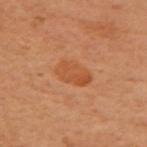Q: Was a biopsy performed?
A: total-body-photography surveillance lesion; no biopsy
Q: Where on the body is the lesion?
A: the arm
Q: What did automated image analysis measure?
A: a lesion area of about 7 mm² and two-axis asymmetry of about 0.25; an automated nevus-likeness rating near 10 out of 100 and a lesion-detection confidence of about 100/100
Q: What is the imaging modality?
A: ~15 mm crop, total-body skin-cancer survey
Q: Who is the patient?
A: female, aged around 60
Q: Lesion size?
A: about 3.5 mm